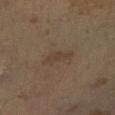No biopsy was performed on this lesion — it was imaged during a full skin examination and was not determined to be concerning. The tile uses cross-polarized illumination. A roughly 15 mm field-of-view crop from a total-body skin photograph. A male patient aged 63 to 67. Longest diameter approximately 3.5 mm. The lesion-visualizer software estimated a shape eccentricity near 0.85 and a symmetry-axis asymmetry near 0.35. And it measured an average lesion color of about L≈38 a*≈12 b*≈25 (CIELAB) and about 5 CIELAB-L* units darker than the surrounding skin. The analysis additionally found peripheral color asymmetry of about 0.5. The software also gave a nevus-likeness score of about 5/100 and a detector confidence of about 100 out of 100 that the crop contains a lesion.